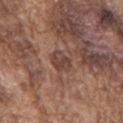Image and clinical context:
The tile uses white-light illumination. The lesion is on the mid back. A male subject aged 73 to 77. Measured at roughly 2.5 mm in maximum diameter. Automated tile analysis of the lesion measured a mean CIELAB color near L≈42 a*≈19 b*≈24, a lesion–skin lightness drop of about 9, and a normalized border contrast of about 7.5. And it measured a detector confidence of about 60 out of 100 that the crop contains a lesion. A close-up tile cropped from a whole-body skin photograph, about 15 mm across.